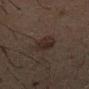  site: back
  image:
    source: total-body photography crop
    field_of_view_mm: 15
  patient:
    sex: male
    age_approx: 50